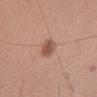Q: Was this lesion biopsied?
A: imaged on a skin check; not biopsied
Q: Lesion size?
A: ≈3.5 mm
Q: Lesion location?
A: the mid back
Q: Automated lesion metrics?
A: an area of roughly 4.5 mm², an eccentricity of roughly 0.9, and a symmetry-axis asymmetry near 0.2; a within-lesion color-variation index near 3.5/10 and a peripheral color-asymmetry measure near 1; a nevus-likeness score of about 95/100 and a lesion-detection confidence of about 100/100
Q: What kind of image is this?
A: total-body-photography crop, ~15 mm field of view
Q: What are the patient's age and sex?
A: male, aged approximately 60